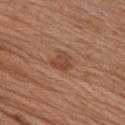The lesion was photographed on a routine skin check and not biopsied; there is no pathology result. From the front of the torso. Approximately 2.5 mm at its widest. A male patient, approximately 60 years of age. A 15 mm close-up extracted from a 3D total-body photography capture. This is a white-light tile.